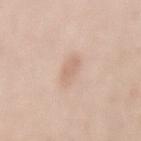Part of a total-body skin-imaging series; this lesion was reviewed on a skin check and was not flagged for biopsy. On the back. The patient is a female in their 50s. A 15 mm close-up tile from a total-body photography series done for melanoma screening.